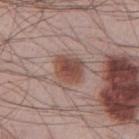Measured at roughly 3.5 mm in maximum diameter.
On the chest.
A male subject, aged around 65.
A 15 mm close-up tile from a total-body photography series done for melanoma screening.
The tile uses white-light illumination.
Automated tile analysis of the lesion measured an outline eccentricity of about 0.5 (0 = round, 1 = elongated) and two-axis asymmetry of about 0.15. It also reported a border-irregularity index near 1.5/10, internal color variation of about 5 on a 0–10 scale, and a peripheral color-asymmetry measure near 1.5. The software also gave a detector confidence of about 100 out of 100 that the crop contains a lesion.